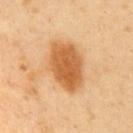notes — total-body-photography surveillance lesion; no biopsy | imaging modality — ~15 mm tile from a whole-body skin photo | patient — male, in their 50s | TBP lesion metrics — a shape eccentricity near 0.75 and a shape-asymmetry score of about 0.2 (0 = symmetric); an average lesion color of about L≈60 a*≈24 b*≈43 (CIELAB), roughly 14 lightness units darker than nearby skin, and a normalized border contrast of about 9 | illumination — cross-polarized | lesion size — ≈6 mm | site — the front of the torso.